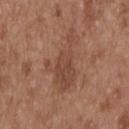– biopsy status — catalogued during a skin exam; not biopsied
– patient — male, roughly 55 years of age
– automated metrics — a color-variation rating of about 3/10; a nevus-likeness score of about 0/100 and a detector confidence of about 100 out of 100 that the crop contains a lesion
– size — ≈7 mm
– image source — total-body-photography crop, ~15 mm field of view
– anatomic site — the upper back